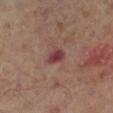The lesion was tiled from a total-body skin photograph and was not biopsied. The total-body-photography lesion software estimated an average lesion color of about L≈38 a*≈24 b*≈19 (CIELAB), roughly 10 lightness units darker than nearby skin, and a normalized lesion–skin contrast near 9. The software also gave border irregularity of about 2 on a 0–10 scale and a peripheral color-asymmetry measure near 0.5. From the right lower leg. Cropped from a total-body skin-imaging series; the visible field is about 15 mm. A male subject in their 70s. The lesion's longest dimension is about 2.5 mm.